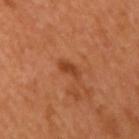biopsy status=no biopsy performed (imaged during a skin exam); image source=~15 mm tile from a whole-body skin photo; tile lighting=cross-polarized; size=≈2.5 mm; anatomic site=the right upper arm; subject=female, aged around 55.